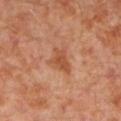Notes:
– notes — no biopsy performed (imaged during a skin exam)
– subject — male, in their 30s
– imaging modality — 15 mm crop, total-body photography
– site — the left lower leg